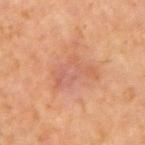Recorded during total-body skin imaging; not selected for excision or biopsy. Captured under cross-polarized illumination. The lesion is on the right upper arm. Measured at roughly 5 mm in maximum diameter. The subject is a male approximately 60 years of age. A roughly 15 mm field-of-view crop from a total-body skin photograph.